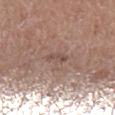| field | value |
|---|---|
| patient | male, aged 48–52 |
| tile lighting | white-light |
| body site | the leg |
| size | ~2.5 mm (longest diameter) |
| imaging modality | total-body-photography crop, ~15 mm field of view |